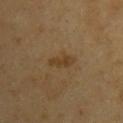Captured during whole-body skin photography for melanoma surveillance; the lesion was not biopsied.
A male subject, aged 63 to 67.
A 15 mm close-up extracted from a 3D total-body photography capture.
About 3.5 mm across.
The lesion is located on the left upper arm.